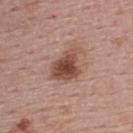No biopsy was performed on this lesion — it was imaged during a full skin examination and was not determined to be concerning. A female patient aged around 55. A 15 mm crop from a total-body photograph taken for skin-cancer surveillance. Captured under white-light illumination. The lesion is located on the upper back.